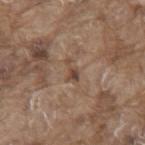Assessment:
Captured during whole-body skin photography for melanoma surveillance; the lesion was not biopsied.
Background:
Cropped from a total-body skin-imaging series; the visible field is about 15 mm. A male patient, aged 78–82. From the back. About 2.5 mm across. The lesion-visualizer software estimated a footprint of about 2.5 mm², an outline eccentricity of about 0.9 (0 = round, 1 = elongated), and a symmetry-axis asymmetry near 0.55. The analysis additionally found a lesion–skin lightness drop of about 9 and a normalized lesion–skin contrast near 7. The analysis additionally found lesion-presence confidence of about 80/100. Captured under white-light illumination.